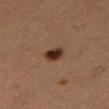Q: Was a biopsy performed?
A: no biopsy performed (imaged during a skin exam)
Q: How was this image acquired?
A: ~15 mm tile from a whole-body skin photo
Q: Illumination type?
A: cross-polarized illumination
Q: Automated lesion metrics?
A: a footprint of about 4.5 mm², an outline eccentricity of about 0.55 (0 = round, 1 = elongated), and a shape-asymmetry score of about 0.2 (0 = symmetric); a lesion color around L≈26 a*≈16 b*≈23 in CIELAB and about 12 CIELAB-L* units darker than the surrounding skin; an automated nevus-likeness rating near 100 out of 100 and a detector confidence of about 100 out of 100 that the crop contains a lesion
Q: Patient demographics?
A: female, aged approximately 40
Q: What is the anatomic site?
A: the leg